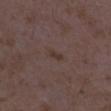Part of a total-body skin-imaging series; this lesion was reviewed on a skin check and was not flagged for biopsy. The tile uses white-light illumination. Measured at roughly 2.5 mm in maximum diameter. A close-up tile cropped from a whole-body skin photograph, about 15 mm across. The lesion is located on the leg. Automated tile analysis of the lesion measured a footprint of about 2.5 mm², an eccentricity of roughly 0.85, and a symmetry-axis asymmetry near 0.3. The subject is a female approximately 35 years of age.